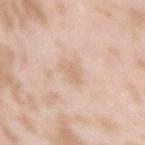Part of a total-body skin-imaging series; this lesion was reviewed on a skin check and was not flagged for biopsy.
Located on the right upper arm.
Approximately 2.5 mm at its widest.
Cropped from a total-body skin-imaging series; the visible field is about 15 mm.
This is a white-light tile.
The patient is a female in their mid- to late 20s.